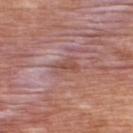workup — total-body-photography surveillance lesion; no biopsy | automated metrics — a footprint of about 5 mm², an outline eccentricity of about 0.8 (0 = round, 1 = elongated), and a shape-asymmetry score of about 0.3 (0 = symmetric); roughly 7 lightness units darker than nearby skin; border irregularity of about 3.5 on a 0–10 scale, internal color variation of about 3 on a 0–10 scale, and a peripheral color-asymmetry measure near 1 | illumination — white-light | site — the upper back | image — ~15 mm crop, total-body skin-cancer survey | subject — male, aged approximately 70.